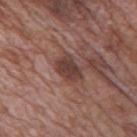follow-up = imaged on a skin check; not biopsied | body site = the mid back | patient = male, approximately 70 years of age | lighting = white-light illumination | size = ≈3.5 mm | imaging modality = ~15 mm crop, total-body skin-cancer survey.